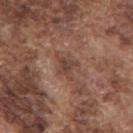Q: Is there a histopathology result?
A: catalogued during a skin exam; not biopsied
Q: What are the patient's age and sex?
A: male, aged 73 to 77
Q: Illumination type?
A: white-light illumination
Q: What is the lesion's diameter?
A: ≈3.5 mm
Q: What did automated image analysis measure?
A: an eccentricity of roughly 0.7 and a symmetry-axis asymmetry near 0.4; a lesion color around L≈43 a*≈20 b*≈25 in CIELAB, about 8 CIELAB-L* units darker than the surrounding skin, and a normalized lesion–skin contrast near 7; a border-irregularity index near 5/10 and internal color variation of about 4 on a 0–10 scale; a nevus-likeness score of about 0/100
Q: What is the imaging modality?
A: ~15 mm tile from a whole-body skin photo
Q: What is the anatomic site?
A: the mid back